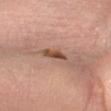Case summary:
• notes · catalogued during a skin exam; not biopsied
• acquisition · 15 mm crop, total-body photography
• location · the right forearm
• automated lesion analysis · an area of roughly 5 mm², an outline eccentricity of about 0.95 (0 = round, 1 = elongated), and a shape-asymmetry score of about 0.3 (0 = symmetric); a color-variation rating of about 2.5/10 and peripheral color asymmetry of about 0.5
• patient · female, roughly 65 years of age
• tile lighting · cross-polarized